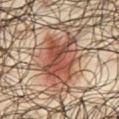Recorded during total-body skin imaging; not selected for excision or biopsy.
A 15 mm close-up tile from a total-body photography series done for melanoma screening.
The lesion's longest dimension is about 6 mm.
A male subject in their mid- to late 60s.
Automated tile analysis of the lesion measured a footprint of about 19 mm² and a symmetry-axis asymmetry near 0.25. And it measured a mean CIELAB color near L≈46 a*≈23 b*≈28, about 12 CIELAB-L* units darker than the surrounding skin, and a lesion-to-skin contrast of about 9.5 (normalized; higher = more distinct). It also reported border irregularity of about 4 on a 0–10 scale, a within-lesion color-variation index near 7.5/10, and peripheral color asymmetry of about 3.
From the chest.
Imaged with cross-polarized lighting.